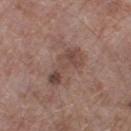biopsy status: no biopsy performed (imaged during a skin exam)
anatomic site: the right lower leg
TBP lesion metrics: a lesion–skin lightness drop of about 9 and a normalized lesion–skin contrast near 7
lighting: white-light
image: total-body-photography crop, ~15 mm field of view
patient: male, approximately 70 years of age